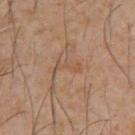Clinical impression: Part of a total-body skin-imaging series; this lesion was reviewed on a skin check and was not flagged for biopsy. Image and clinical context: Automated image analysis of the tile measured an area of roughly 3 mm² and two-axis asymmetry of about 0.4. The software also gave roughly 5 lightness units darker than nearby skin. The software also gave an automated nevus-likeness rating near 0 out of 100 and a detector confidence of about 95 out of 100 that the crop contains a lesion. Measured at roughly 3.5 mm in maximum diameter. Captured under cross-polarized illumination. A 15 mm crop from a total-body photograph taken for skin-cancer surveillance. The lesion is located on the front of the torso. A male patient about 60 years old.